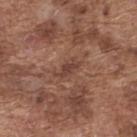Q: Was this lesion biopsied?
A: imaged on a skin check; not biopsied
Q: Who is the patient?
A: male, aged around 75
Q: What kind of image is this?
A: 15 mm crop, total-body photography
Q: Lesion size?
A: ≈3.5 mm
Q: Where on the body is the lesion?
A: the upper back
Q: How was the tile lit?
A: white-light illumination